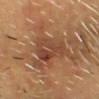Q: Was this lesion biopsied?
A: imaged on a skin check; not biopsied
Q: How was this image acquired?
A: ~15 mm tile from a whole-body skin photo
Q: Illumination type?
A: cross-polarized
Q: What are the patient's age and sex?
A: male, approximately 65 years of age
Q: Lesion location?
A: the front of the torso
Q: Lesion size?
A: ≈6 mm